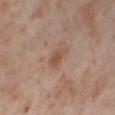The lesion was photographed on a routine skin check and not biopsied; there is no pathology result. Imaged with cross-polarized lighting. A female subject approximately 55 years of age. The recorded lesion diameter is about 3 mm. From the right thigh. A region of skin cropped from a whole-body photographic capture, roughly 15 mm wide.